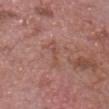Notes:
* biopsy status: imaged on a skin check; not biopsied
* subject: male, aged 73 to 77
* lesion size: about 4 mm
* location: the chest
* image source: ~15 mm tile from a whole-body skin photo
* automated metrics: a border-irregularity rating of about 5.5/10 and radial color variation of about 0; a nevus-likeness score of about 0/100 and a detector confidence of about 95 out of 100 that the crop contains a lesion